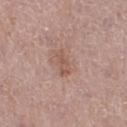<case>
  <biopsy_status>not biopsied; imaged during a skin examination</biopsy_status>
  <lesion_size>
    <long_diameter_mm_approx>3.0</long_diameter_mm_approx>
  </lesion_size>
  <image>
    <source>total-body photography crop</source>
    <field_of_view_mm>15</field_of_view_mm>
  </image>
  <patient>
    <sex>female</sex>
    <age_approx>40</age_approx>
  </patient>
  <site>left lower leg</site>
</case>